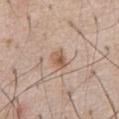Q: Is there a histopathology result?
A: catalogued during a skin exam; not biopsied
Q: What are the patient's age and sex?
A: male, roughly 60 years of age
Q: How was the tile lit?
A: white-light illumination
Q: What did automated image analysis measure?
A: an average lesion color of about L≈57 a*≈18 b*≈30 (CIELAB) and a normalized lesion–skin contrast near 7.5
Q: What is the imaging modality?
A: 15 mm crop, total-body photography
Q: Lesion location?
A: the chest
Q: How large is the lesion?
A: about 2.5 mm